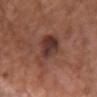{
  "biopsy_status": "not biopsied; imaged during a skin examination",
  "automated_metrics": {
    "area_mm2_approx": 12.0,
    "eccentricity": 0.65,
    "shape_asymmetry": 0.35,
    "vs_skin_darker_L": 10.0,
    "vs_skin_contrast_norm": 9.0,
    "border_irregularity_0_10": 4.0,
    "color_variation_0_10": 6.5,
    "nevus_likeness_0_100": 20
  },
  "lesion_size": {
    "long_diameter_mm_approx": 4.5
  },
  "image": {
    "source": "total-body photography crop",
    "field_of_view_mm": 15
  },
  "lighting": "white-light",
  "site": "chest",
  "patient": {
    "sex": "female",
    "age_approx": 75
  }
}